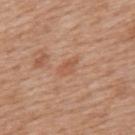biopsy_status: not biopsied; imaged during a skin examination
patient:
  sex: male
  age_approx: 60
lesion_size:
  long_diameter_mm_approx: 3.0
automated_metrics:
  border_irregularity_0_10: 2.5
  color_variation_0_10: 1.0
  peripheral_color_asymmetry: 0.0
  nevus_likeness_0_100: 0
  lesion_detection_confidence_0_100: 100
lighting: white-light
site: upper back
image:
  source: total-body photography crop
  field_of_view_mm: 15